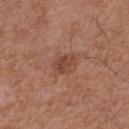workup: no biopsy performed (imaged during a skin exam) | acquisition: ~15 mm tile from a whole-body skin photo | location: the right thigh | image-analysis metrics: an area of roughly 5 mm², an eccentricity of roughly 0.55, and a shape-asymmetry score of about 0.2 (0 = symmetric); a mean CIELAB color near L≈45 a*≈23 b*≈28 | lighting: white-light | patient: male, aged around 75 | lesion size: ≈2.5 mm.